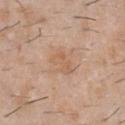Notes:
– biopsy status: catalogued during a skin exam; not biopsied
– subject: male, aged 28–32
– lighting: white-light
– site: the chest
– imaging modality: ~15 mm crop, total-body skin-cancer survey
– size: ~3.5 mm (longest diameter)